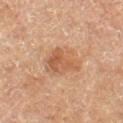Case summary:
– workup · no biopsy performed (imaged during a skin exam)
– patient · female, in their mid-50s
– lesion size · ≈4.5 mm
– tile lighting · cross-polarized
– site · the left thigh
– automated metrics · a lesion-detection confidence of about 100/100
– imaging modality · 15 mm crop, total-body photography